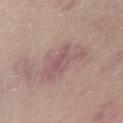Recorded during total-body skin imaging; not selected for excision or biopsy. Approximately 6 mm at its widest. This is a white-light tile. A female subject aged approximately 60. A 15 mm close-up extracted from a 3D total-body photography capture. The lesion is on the chest.